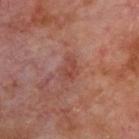follow-up=catalogued during a skin exam; not biopsied | location=the back | patient=male, about 70 years old | imaging modality=total-body-photography crop, ~15 mm field of view | lesion diameter=about 2.5 mm.